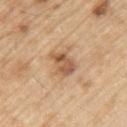Clinical impression:
Recorded during total-body skin imaging; not selected for excision or biopsy.
Clinical summary:
From the arm. Approximately 3.5 mm at its widest. Cropped from a total-body skin-imaging series; the visible field is about 15 mm. A male subject, about 70 years old.